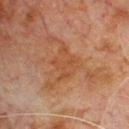follow-up: total-body-photography surveillance lesion; no biopsy | size: ≈4 mm | automated metrics: a symmetry-axis asymmetry near 0.45; radial color variation of about 1; an automated nevus-likeness rating near 0 out of 100 and a detector confidence of about 100 out of 100 that the crop contains a lesion | image: 15 mm crop, total-body photography | tile lighting: cross-polarized illumination | body site: the front of the torso | patient: male, aged around 80.